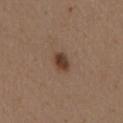{
  "biopsy_status": "not biopsied; imaged during a skin examination",
  "patient": {
    "sex": "female",
    "age_approx": 40
  },
  "automated_metrics": {
    "cielab_L": 39,
    "cielab_a": 18,
    "cielab_b": 27,
    "vs_skin_darker_L": 12.0
  },
  "site": "mid back",
  "lesion_size": {
    "long_diameter_mm_approx": 2.5
  },
  "image": {
    "source": "total-body photography crop",
    "field_of_view_mm": 15
  },
  "lighting": "white-light"
}